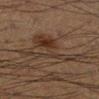Part of a total-body skin-imaging series; this lesion was reviewed on a skin check and was not flagged for biopsy.
Measured at roughly 8 mm in maximum diameter.
This is a cross-polarized tile.
A roughly 15 mm field-of-view crop from a total-body skin photograph.
The lesion is on the right lower leg.
A male subject, aged approximately 55.